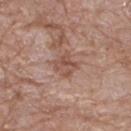Assessment:
Captured during whole-body skin photography for melanoma surveillance; the lesion was not biopsied.
Acquisition and patient details:
From the leg. A 15 mm close-up tile from a total-body photography series done for melanoma screening. A male patient, roughly 80 years of age.